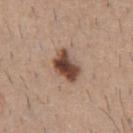A close-up tile cropped from a whole-body skin photograph, about 15 mm across. An algorithmic analysis of the crop reported a footprint of about 8.5 mm² and an eccentricity of roughly 0.75. And it measured a border-irregularity index near 2.5/10 and radial color variation of about 2. The lesion is on the chest. This is a white-light tile. A male subject roughly 60 years of age.